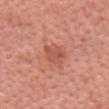No biopsy was performed on this lesion — it was imaged during a full skin examination and was not determined to be concerning. The lesion is on the head or neck. A female subject roughly 50 years of age. Captured under white-light illumination. A close-up tile cropped from a whole-body skin photograph, about 15 mm across.